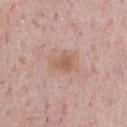– notes · no biopsy performed (imaged during a skin exam)
– patient · male, aged 73–77
– location · the chest
– imaging modality · ~15 mm tile from a whole-body skin photo